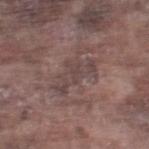Q: Was this lesion biopsied?
A: no biopsy performed (imaged during a skin exam)
Q: Illumination type?
A: white-light illumination
Q: How was this image acquired?
A: ~15 mm tile from a whole-body skin photo
Q: Who is the patient?
A: male, about 75 years old
Q: Lesion size?
A: about 5.5 mm
Q: What did automated image analysis measure?
A: a border-irregularity index near 7/10, a color-variation rating of about 2/10, and a peripheral color-asymmetry measure near 0.5
Q: What is the anatomic site?
A: the right thigh